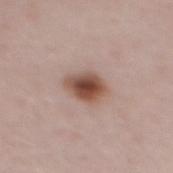The lesion is located on the mid back. This is a white-light tile. A region of skin cropped from a whole-body photographic capture, roughly 15 mm wide. A female subject, aged around 50. Longest diameter approximately 4 mm.